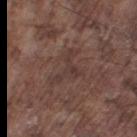Recorded during total-body skin imaging; not selected for excision or biopsy. On the right thigh. A male subject aged 73–77. An algorithmic analysis of the crop reported a lesion color around L≈36 a*≈16 b*≈20 in CIELAB and roughly 6 lightness units darker than nearby skin. It also reported a border-irregularity rating of about 9.5/10, a color-variation rating of about 2.5/10, and radial color variation of about 0.5. Imaged with white-light lighting. Cropped from a total-body skin-imaging series; the visible field is about 15 mm.